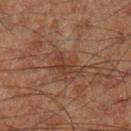Notes:
- biopsy status · imaged on a skin check; not biopsied
- anatomic site · the left lower leg
- diameter · about 3.5 mm
- illumination · cross-polarized illumination
- image · total-body-photography crop, ~15 mm field of view
- subject · male, in their 60s